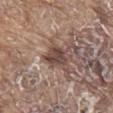Recorded during total-body skin imaging; not selected for excision or biopsy. Imaged with white-light lighting. This image is a 15 mm lesion crop taken from a total-body photograph. On the mid back. A male subject aged around 80.